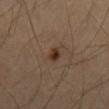| field | value |
|---|---|
| follow-up | catalogued during a skin exam; not biopsied |
| subject | male, approximately 65 years of age |
| lesion size | ≈2 mm |
| acquisition | 15 mm crop, total-body photography |
| site | the mid back |
| lighting | cross-polarized |